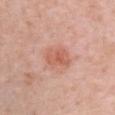This lesion was catalogued during total-body skin photography and was not selected for biopsy. The lesion is located on the chest. A 15 mm close-up tile from a total-body photography series done for melanoma screening. A female patient about 40 years old. Approximately 3.5 mm at its widest.